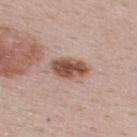biopsy_status: not biopsied; imaged during a skin examination
lesion_size:
  long_diameter_mm_approx: 4.0
lighting: white-light
image:
  source: total-body photography crop
  field_of_view_mm: 15
site: upper back
patient:
  sex: male
  age_approx: 60
automated_metrics:
  area_mm2_approx: 8.0
  eccentricity: 0.8
  shape_asymmetry: 0.15
  cielab_L: 51
  cielab_a: 20
  cielab_b: 27